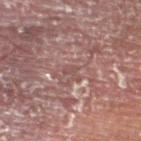notes: no biopsy performed (imaged during a skin exam) | image source: total-body-photography crop, ~15 mm field of view | subject: male, aged 53–57 | location: the left lower leg.